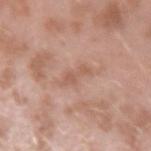biopsy status=imaged on a skin check; not biopsied